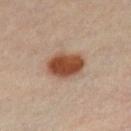workup = imaged on a skin check; not biopsied | TBP lesion metrics = an automated nevus-likeness rating near 100 out of 100 and a detector confidence of about 100 out of 100 that the crop contains a lesion | subject = male, aged around 35 | lesion size = ~4.5 mm (longest diameter) | tile lighting = cross-polarized illumination | imaging modality = total-body-photography crop, ~15 mm field of view | site = the chest.